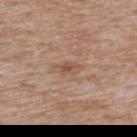Clinical summary: Longest diameter approximately 2.5 mm. A roughly 15 mm field-of-view crop from a total-body skin photograph. The lesion is located on the upper back. A male patient, in their mid-60s. This is a white-light tile.